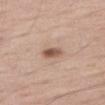Case summary:
– biopsy status: total-body-photography surveillance lesion; no biopsy
– image source: total-body-photography crop, ~15 mm field of view
– image-analysis metrics: an area of roughly 4 mm² and a shape eccentricity near 0.75
– subject: male, aged approximately 65
– body site: the right thigh
– illumination: white-light illumination